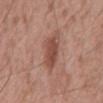Clinical impression:
This lesion was catalogued during total-body skin photography and was not selected for biopsy.
Image and clinical context:
This image is a 15 mm lesion crop taken from a total-body photograph. Imaged with white-light lighting. The lesion is on the mid back. The subject is a male about 60 years old.